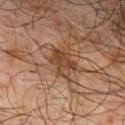follow-up: imaged on a skin check; not biopsied
image: total-body-photography crop, ~15 mm field of view
body site: the upper back
patient: male, about 65 years old